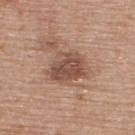Acquisition and patient details: Located on the upper back. A female patient, aged around 60. A roughly 15 mm field-of-view crop from a total-body skin photograph.